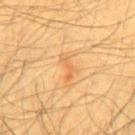notes: no biopsy performed (imaged during a skin exam)
TBP lesion metrics: a footprint of about 3 mm², an eccentricity of roughly 0.95, and a symmetry-axis asymmetry near 0.65; border irregularity of about 7 on a 0–10 scale and internal color variation of about 0 on a 0–10 scale; an automated nevus-likeness rating near 0 out of 100 and a lesion-detection confidence of about 100/100
site: the chest
subject: male, aged approximately 60
tile lighting: cross-polarized illumination
lesion size: ~3 mm (longest diameter)
imaging modality: total-body-photography crop, ~15 mm field of view